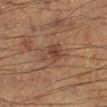Assessment:
Imaged during a routine full-body skin examination; the lesion was not biopsied and no histopathology is available.
Clinical summary:
This image is a 15 mm lesion crop taken from a total-body photograph. A male subject, aged approximately 55. On the leg. Imaged with cross-polarized lighting.